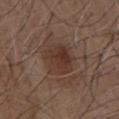notes — total-body-photography surveillance lesion; no biopsy | image source — ~15 mm tile from a whole-body skin photo | image-analysis metrics — a lesion area of about 12 mm², a shape eccentricity near 0.6, and a shape-asymmetry score of about 0.2 (0 = symmetric) | lesion size — ≈4.5 mm | body site — the chest | illumination — white-light | patient — male, in their mid- to late 70s.